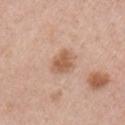biopsy_status: not biopsied; imaged during a skin examination
lesion_size:
  long_diameter_mm_approx: 3.0
automated_metrics:
  cielab_L: 58
  cielab_a: 21
  cielab_b: 32
  vs_skin_darker_L: 11.0
  vs_skin_contrast_norm: 7.5
  border_irregularity_0_10: 2.5
  color_variation_0_10: 2.0
  peripheral_color_asymmetry: 0.5
  nevus_likeness_0_100: 65
  lesion_detection_confidence_0_100: 100
lighting: white-light
patient:
  sex: female
  age_approx: 50
site: left upper arm
image:
  source: total-body photography crop
  field_of_view_mm: 15